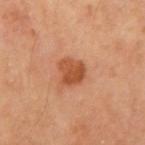Q: Was this lesion biopsied?
A: catalogued during a skin exam; not biopsied
Q: Patient demographics?
A: male, aged 63 to 67
Q: Where on the body is the lesion?
A: the right upper arm
Q: How was this image acquired?
A: total-body-photography crop, ~15 mm field of view